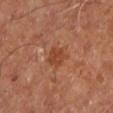Findings:
– workup: total-body-photography surveillance lesion; no biopsy
– acquisition: total-body-photography crop, ~15 mm field of view
– patient: male, aged approximately 60
– body site: the right leg
– lighting: cross-polarized illumination
– TBP lesion metrics: a lesion color around L≈42 a*≈26 b*≈32 in CIELAB, a lesion–skin lightness drop of about 8, and a lesion-to-skin contrast of about 6.5 (normalized; higher = more distinct); border irregularity of about 2.5 on a 0–10 scale, internal color variation of about 2 on a 0–10 scale, and peripheral color asymmetry of about 1